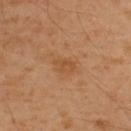No biopsy was performed on this lesion — it was imaged during a full skin examination and was not determined to be concerning.